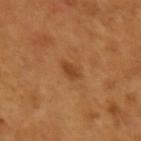Part of a total-body skin-imaging series; this lesion was reviewed on a skin check and was not flagged for biopsy. Imaged with cross-polarized lighting. A female subject in their mid-50s. The lesion's longest dimension is about 2.5 mm. Automated image analysis of the tile measured a lesion area of about 2.5 mm², an outline eccentricity of about 0.8 (0 = round, 1 = elongated), and a symmetry-axis asymmetry near 0.25. It also reported a border-irregularity index near 2.5/10, internal color variation of about 1 on a 0–10 scale, and peripheral color asymmetry of about 0.5. The lesion is located on the left forearm. This image is a 15 mm lesion crop taken from a total-body photograph.